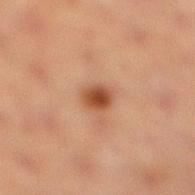The lesion was tiled from a total-body skin photograph and was not biopsied. Approximately 2.5 mm at its widest. A close-up tile cropped from a whole-body skin photograph, about 15 mm across. On the right lower leg. The patient is a female approximately 40 years of age.